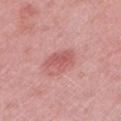notes: total-body-photography surveillance lesion; no biopsy
lesion diameter: about 4 mm
automated lesion analysis: an area of roughly 6.5 mm², an outline eccentricity of about 0.8 (0 = round, 1 = elongated), and a shape-asymmetry score of about 0.3 (0 = symmetric); a mean CIELAB color near L≈57 a*≈29 b*≈25, about 9 CIELAB-L* units darker than the surrounding skin, and a lesion-to-skin contrast of about 6 (normalized; higher = more distinct); a color-variation rating of about 2/10 and radial color variation of about 0.5; lesion-presence confidence of about 100/100
imaging modality: total-body-photography crop, ~15 mm field of view
location: the left lower leg
tile lighting: white-light
patient: female, aged 38–42